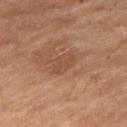The lesion was photographed on a routine skin check and not biopsied; there is no pathology result. Located on the leg. The subject is a male roughly 60 years of age. A close-up tile cropped from a whole-body skin photograph, about 15 mm across. An algorithmic analysis of the crop reported a footprint of about 5 mm², a shape eccentricity near 0.8, and a symmetry-axis asymmetry near 0.3. And it measured a mean CIELAB color near L≈46 a*≈20 b*≈30, about 6 CIELAB-L* units darker than the surrounding skin, and a lesion-to-skin contrast of about 5 (normalized; higher = more distinct). Imaged with cross-polarized lighting.